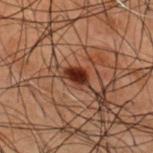| key | value |
|---|---|
| follow-up | no biopsy performed (imaged during a skin exam) |
| lesion size | ≈3 mm |
| automated metrics | an area of roughly 4.5 mm², an eccentricity of roughly 0.8, and two-axis asymmetry of about 0.25; a normalized lesion–skin contrast near 13; a border-irregularity index near 2.5/10, a within-lesion color-variation index near 2.5/10, and peripheral color asymmetry of about 1 |
| image source | 15 mm crop, total-body photography |
| anatomic site | the mid back |
| subject | male, roughly 50 years of age |